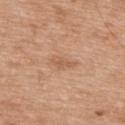Recorded during total-body skin imaging; not selected for excision or biopsy. A male subject, aged 48–52. A 15 mm close-up tile from a total-body photography series done for melanoma screening. Measured at roughly 3 mm in maximum diameter. Located on the upper back. This is a white-light tile.